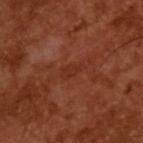Findings:
* biopsy status — total-body-photography surveillance lesion; no biopsy
* subject — male, roughly 65 years of age
* imaging modality — ~15 mm crop, total-body skin-cancer survey